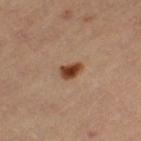Recorded during total-body skin imaging; not selected for excision or biopsy.
A female subject about 45 years old.
The lesion is on the left thigh.
Captured under cross-polarized illumination.
This image is a 15 mm lesion crop taken from a total-body photograph.